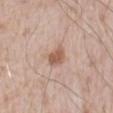{
  "biopsy_status": "not biopsied; imaged during a skin examination",
  "automated_metrics": {
    "vs_skin_darker_L": 11.0,
    "nevus_likeness_0_100": 70,
    "lesion_detection_confidence_0_100": 100
  },
  "lighting": "white-light",
  "site": "front of the torso",
  "image": {
    "source": "total-body photography crop",
    "field_of_view_mm": 15
  },
  "lesion_size": {
    "long_diameter_mm_approx": 3.0
  },
  "patient": {
    "sex": "male",
    "age_approx": 80
  }
}